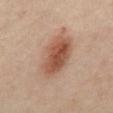biopsy status = imaged on a skin check; not biopsied | lesion size = ~6 mm (longest diameter) | imaging modality = 15 mm crop, total-body photography | patient = male, aged around 65 | location = the mid back | illumination = cross-polarized.